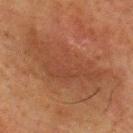The lesion is located on the upper back.
A close-up tile cropped from a whole-body skin photograph, about 15 mm across.
The patient is a male about 60 years old.